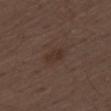follow-up — catalogued during a skin exam; not biopsied | tile lighting — white-light | site — the left thigh | image source — total-body-photography crop, ~15 mm field of view | automated lesion analysis — an average lesion color of about L≈31 a*≈15 b*≈22 (CIELAB); border irregularity of about 2 on a 0–10 scale and a color-variation rating of about 2/10 | patient — male, aged 68 to 72.